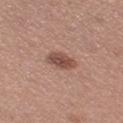Assessment:
Recorded during total-body skin imaging; not selected for excision or biopsy.
Context:
The patient is a female roughly 40 years of age. Measured at roughly 3.5 mm in maximum diameter. Captured under white-light illumination. The lesion is on the left thigh. A lesion tile, about 15 mm wide, cut from a 3D total-body photograph. The lesion-visualizer software estimated a lesion color around L≈49 a*≈21 b*≈25 in CIELAB and a normalized border contrast of about 8.5.